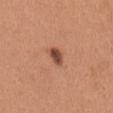This lesion was catalogued during total-body skin photography and was not selected for biopsy.
Cropped from a total-body skin-imaging series; the visible field is about 15 mm.
The lesion is on the back.
The lesion's longest dimension is about 2.5 mm.
The patient is a female aged around 30.
The tile uses white-light illumination.